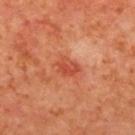biopsy_status: not biopsied; imaged during a skin examination
patient:
  sex: female
  age_approx: 40
lesion_size:
  long_diameter_mm_approx: 3.0
image:
  source: total-body photography crop
  field_of_view_mm: 15
automated_metrics:
  area_mm2_approx: 5.5
  shape_asymmetry: 0.3
  border_irregularity_0_10: 2.5
  color_variation_0_10: 4.0
  peripheral_color_asymmetry: 1.5
  nevus_likeness_0_100: 0
site: upper back